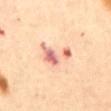The lesion was photographed on a routine skin check and not biopsied; there is no pathology result. The lesion is located on the abdomen. Cropped from a whole-body photographic skin survey; the tile spans about 15 mm. The subject is a female about 65 years old.